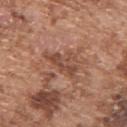Captured during whole-body skin photography for melanoma surveillance; the lesion was not biopsied. A region of skin cropped from a whole-body photographic capture, roughly 15 mm wide. This is a white-light tile. Automated image analysis of the tile measured a lesion color around L≈47 a*≈22 b*≈29 in CIELAB, a lesion–skin lightness drop of about 9, and a normalized border contrast of about 6.5. It also reported a border-irregularity index near 7/10, internal color variation of about 3 on a 0–10 scale, and a peripheral color-asymmetry measure near 1. It also reported a nevus-likeness score of about 0/100 and lesion-presence confidence of about 80/100. Located on the upper back. The patient is a male aged 73 to 77. Measured at roughly 5 mm in maximum diameter.